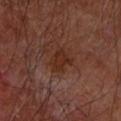{"biopsy_status": "not biopsied; imaged during a skin examination", "patient": {"sex": "male", "age_approx": 65}, "lighting": "cross-polarized", "image": {"source": "total-body photography crop", "field_of_view_mm": 15}, "site": "left forearm", "lesion_size": {"long_diameter_mm_approx": 3.0}}